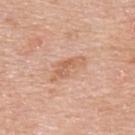Impression:
No biopsy was performed on this lesion — it was imaged during a full skin examination and was not determined to be concerning.
Clinical summary:
This image is a 15 mm lesion crop taken from a total-body photograph. A male subject in their 80s. The lesion is on the upper back.